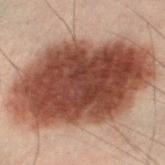Notes:
– follow-up — total-body-photography surveillance lesion; no biopsy
– image — total-body-photography crop, ~15 mm field of view
– diameter — ≈13.5 mm
– patient — male, aged around 50
– tile lighting — cross-polarized illumination
– site — the left lower leg